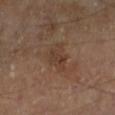notes: total-body-photography surveillance lesion; no biopsy | subject: male, aged 68–72 | diameter: ~4 mm (longest diameter) | location: the leg | image-analysis metrics: internal color variation of about 2.5 on a 0–10 scale and peripheral color asymmetry of about 1; a classifier nevus-likeness of about 0/100 | illumination: cross-polarized illumination | image: total-body-photography crop, ~15 mm field of view.